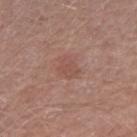The lesion was photographed on a routine skin check and not biopsied; there is no pathology result. The total-body-photography lesion software estimated a footprint of about 5 mm², an eccentricity of roughly 0.7, and a symmetry-axis asymmetry near 0.25. And it measured a lesion color around L≈51 a*≈21 b*≈25 in CIELAB and a normalized border contrast of about 4.5. It also reported border irregularity of about 2.5 on a 0–10 scale, a color-variation rating of about 1.5/10, and a peripheral color-asymmetry measure near 0.5. About 3 mm across. The tile uses white-light illumination. Cropped from a whole-body photographic skin survey; the tile spans about 15 mm. The patient is a male aged approximately 60. From the right forearm.